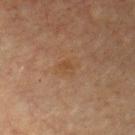{
  "biopsy_status": "not biopsied; imaged during a skin examination",
  "lesion_size": {
    "long_diameter_mm_approx": 2.5
  },
  "lighting": "cross-polarized",
  "patient": {
    "sex": "male",
    "age_approx": 65
  },
  "site": "back",
  "automated_metrics": {
    "area_mm2_approx": 4.0,
    "eccentricity": 0.75,
    "shape_asymmetry": 0.25,
    "cielab_L": 43,
    "cielab_a": 17,
    "cielab_b": 31,
    "vs_skin_darker_L": 4.0,
    "vs_skin_contrast_norm": 5.0,
    "peripheral_color_asymmetry": 0.5
  },
  "image": {
    "source": "total-body photography crop",
    "field_of_view_mm": 15
  }
}